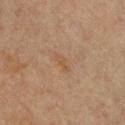notes: no biopsy performed (imaged during a skin exam) | size: ~2.5 mm (longest diameter) | tile lighting: cross-polarized | imaging modality: ~15 mm tile from a whole-body skin photo | subject: female, aged 43 to 47 | automated lesion analysis: an outline eccentricity of about 0.9 (0 = round, 1 = elongated) and two-axis asymmetry of about 0.2; a mean CIELAB color near L≈45 a*≈16 b*≈30 and a lesion–skin lightness drop of about 5; a classifier nevus-likeness of about 0/100 and a detector confidence of about 100 out of 100 that the crop contains a lesion | body site: the chest.